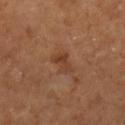Assessment: The lesion was photographed on a routine skin check and not biopsied; there is no pathology result. Acquisition and patient details: The total-body-photography lesion software estimated a lesion area of about 4.5 mm², an eccentricity of roughly 0.85, and a shape-asymmetry score of about 0.45 (0 = symmetric). The analysis additionally found a mean CIELAB color near L≈39 a*≈21 b*≈30, roughly 7 lightness units darker than nearby skin, and a normalized lesion–skin contrast near 6. And it measured a border-irregularity rating of about 4.5/10, a color-variation rating of about 3.5/10, and radial color variation of about 1. It also reported a classifier nevus-likeness of about 0/100. The lesion is on the right lower leg. A lesion tile, about 15 mm wide, cut from a 3D total-body photograph. A female patient, roughly 65 years of age. Imaged with cross-polarized lighting. About 3.5 mm across.